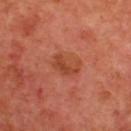workup — catalogued during a skin exam; not biopsied | patient — male, aged approximately 65 | TBP lesion metrics — a footprint of about 6 mm² and an eccentricity of roughly 0.85; a mean CIELAB color near L≈44 a*≈29 b*≈35, about 7 CIELAB-L* units darker than the surrounding skin, and a normalized border contrast of about 6.5; a border-irregularity index near 4/10, internal color variation of about 2.5 on a 0–10 scale, and peripheral color asymmetry of about 1; a detector confidence of about 100 out of 100 that the crop contains a lesion | image source — total-body-photography crop, ~15 mm field of view | lighting — cross-polarized | location — the upper back | diameter — about 3.5 mm.